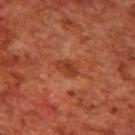notes = total-body-photography surveillance lesion; no biopsy | lighting = cross-polarized | TBP lesion metrics = a shape eccentricity near 0.8; a border-irregularity rating of about 2.5/10, a within-lesion color-variation index near 2/10, and radial color variation of about 0.5 | imaging modality = total-body-photography crop, ~15 mm field of view | patient = male, roughly 70 years of age | anatomic site = the upper back.